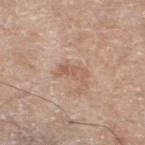follow-up: imaged on a skin check; not biopsied | lesion diameter: ≈3.5 mm | image source: total-body-photography crop, ~15 mm field of view | subject: female, in their mid- to late 70s | anatomic site: the leg.